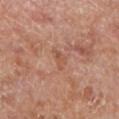Cropped from a whole-body photographic skin survey; the tile spans about 15 mm.
Captured under cross-polarized illumination.
Measured at roughly 3 mm in maximum diameter.
Automated tile analysis of the lesion measured radial color variation of about 0.5.
From the left lower leg.
A male subject, aged 68 to 72.